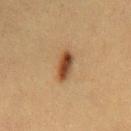biopsy status: catalogued during a skin exam; not biopsied | automated metrics: a mean CIELAB color near L≈41 a*≈19 b*≈32, about 13 CIELAB-L* units darker than the surrounding skin, and a lesion-to-skin contrast of about 10.5 (normalized; higher = more distinct); a nevus-likeness score of about 100/100 and a lesion-detection confidence of about 100/100 | site: the mid back | imaging modality: ~15 mm crop, total-body skin-cancer survey | size: about 4 mm | subject: female, aged 38–42.